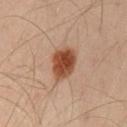Q: What kind of image is this?
A: ~15 mm tile from a whole-body skin photo
Q: What lighting was used for the tile?
A: cross-polarized illumination
Q: Who is the patient?
A: male, aged around 60
Q: What is the anatomic site?
A: the front of the torso